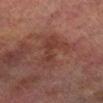A lesion tile, about 15 mm wide, cut from a 3D total-body photograph. The recorded lesion diameter is about 5.5 mm. On the right lower leg. The patient is a male about 75 years old. Imaged with cross-polarized lighting.